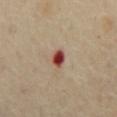Impression:
This lesion was catalogued during total-body skin photography and was not selected for biopsy.
Context:
The lesion is on the abdomen. A roughly 15 mm field-of-view crop from a total-body skin photograph. A female subject, approximately 70 years of age. The lesion's longest dimension is about 2.5 mm.